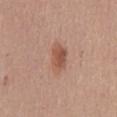This lesion was catalogued during total-body skin photography and was not selected for biopsy.
Measured at roughly 4 mm in maximum diameter.
Cropped from a whole-body photographic skin survey; the tile spans about 15 mm.
The lesion-visualizer software estimated a footprint of about 6.5 mm² and a shape eccentricity near 0.85. It also reported a border-irregularity rating of about 2.5/10, a within-lesion color-variation index near 3.5/10, and radial color variation of about 1. The software also gave a nevus-likeness score of about 90/100.
From the mid back.
Imaged with white-light lighting.
A female patient approximately 60 years of age.